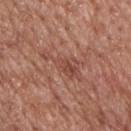| feature | finding |
|---|---|
| notes | total-body-photography surveillance lesion; no biopsy |
| imaging modality | total-body-photography crop, ~15 mm field of view |
| patient | male, in their 60s |
| body site | the upper back |
| automated metrics | a lesion area of about 8.5 mm², an outline eccentricity of about 0.95 (0 = round, 1 = elongated), and a shape-asymmetry score of about 0.55 (0 = symmetric); border irregularity of about 8 on a 0–10 scale and a within-lesion color-variation index near 4.5/10 |
| lesion size | ~6 mm (longest diameter) |